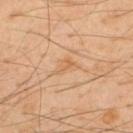Impression: No biopsy was performed on this lesion — it was imaged during a full skin examination and was not determined to be concerning. Context: The subject is a male aged approximately 50. A 15 mm close-up extracted from a 3D total-body photography capture. Located on the upper back. Longest diameter approximately 3 mm. The tile uses cross-polarized illumination.